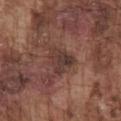This lesion was catalogued during total-body skin photography and was not selected for biopsy. A lesion tile, about 15 mm wide, cut from a 3D total-body photograph. Imaged with white-light lighting. Approximately 4 mm at its widest. From the chest. A male patient, aged 73 to 77. An algorithmic analysis of the crop reported an area of roughly 8.5 mm², an eccentricity of roughly 0.55, and a shape-asymmetry score of about 0.4 (0 = symmetric). The software also gave a border-irregularity index near 3.5/10, internal color variation of about 4.5 on a 0–10 scale, and a peripheral color-asymmetry measure near 1.5. And it measured a classifier nevus-likeness of about 20/100 and a detector confidence of about 95 out of 100 that the crop contains a lesion.